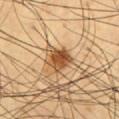follow-up: no biopsy performed (imaged during a skin exam) | TBP lesion metrics: border irregularity of about 2.5 on a 0–10 scale, a color-variation rating of about 5.5/10, and a peripheral color-asymmetry measure near 2; an automated nevus-likeness rating near 95 out of 100 and lesion-presence confidence of about 100/100 | imaging modality: 15 mm crop, total-body photography | body site: the chest | patient: male, aged 58–62 | size: about 3 mm | illumination: cross-polarized.